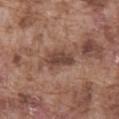biopsy status: catalogued during a skin exam; not biopsied
subject: male, roughly 75 years of age
lesion diameter: about 3.5 mm
body site: the abdomen
illumination: white-light
acquisition: total-body-photography crop, ~15 mm field of view